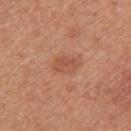No biopsy was performed on this lesion — it was imaged during a full skin examination and was not determined to be concerning.
From the arm.
The recorded lesion diameter is about 3.5 mm.
A male patient in their 30s.
The tile uses white-light illumination.
A roughly 15 mm field-of-view crop from a total-body skin photograph.